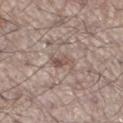biopsy status = catalogued during a skin exam; not biopsied
patient = male, approximately 55 years of age
site = the left lower leg
diameter = ≈2.5 mm
image source = ~15 mm tile from a whole-body skin photo